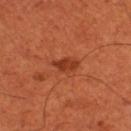biopsy status: no biopsy performed (imaged during a skin exam)
patient: male, about 50 years old
acquisition: total-body-photography crop, ~15 mm field of view
site: the left thigh
automated lesion analysis: an area of roughly 4 mm², an eccentricity of roughly 0.8, and a shape-asymmetry score of about 0.25 (0 = symmetric); a border-irregularity rating of about 2.5/10, a color-variation rating of about 2/10, and peripheral color asymmetry of about 0.5
lighting: cross-polarized
size: about 3 mm